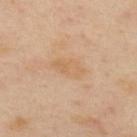This lesion was catalogued during total-body skin photography and was not selected for biopsy. The recorded lesion diameter is about 4 mm. Captured under cross-polarized illumination. Located on the upper back. A 15 mm crop from a total-body photograph taken for skin-cancer surveillance. An algorithmic analysis of the crop reported a lesion color around L≈64 a*≈18 b*≈37 in CIELAB, a lesion–skin lightness drop of about 6, and a normalized border contrast of about 5. It also reported a classifier nevus-likeness of about 0/100. A female subject roughly 40 years of age.